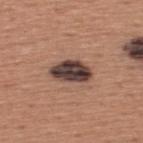workup=no biopsy performed (imaged during a skin exam); diameter=~4.5 mm (longest diameter); patient=male, aged approximately 45; tile lighting=white-light; image=total-body-photography crop, ~15 mm field of view; anatomic site=the back.